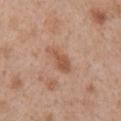Clinical impression:
Part of a total-body skin-imaging series; this lesion was reviewed on a skin check and was not flagged for biopsy.
Image and clinical context:
The tile uses white-light illumination. A region of skin cropped from a whole-body photographic capture, roughly 15 mm wide. A female patient aged 38–42. The lesion's longest dimension is about 3.5 mm. On the right upper arm. An algorithmic analysis of the crop reported an area of roughly 5 mm² and a shape-asymmetry score of about 0.3 (0 = symmetric). It also reported an average lesion color of about L≈54 a*≈21 b*≈32 (CIELAB), about 9 CIELAB-L* units darker than the surrounding skin, and a lesion-to-skin contrast of about 6.5 (normalized; higher = more distinct).